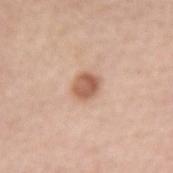Clinical impression:
Recorded during total-body skin imaging; not selected for excision or biopsy.
Image and clinical context:
Longest diameter approximately 2.5 mm. The lesion is located on the right forearm. A lesion tile, about 15 mm wide, cut from a 3D total-body photograph. The tile uses white-light illumination. The patient is a female aged 58–62.